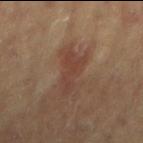Q: Was this lesion biopsied?
A: total-body-photography surveillance lesion; no biopsy
Q: Patient demographics?
A: female, roughly 80 years of age
Q: What is the imaging modality?
A: ~15 mm crop, total-body skin-cancer survey
Q: Lesion location?
A: the left arm